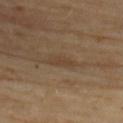Imaged during a routine full-body skin examination; the lesion was not biopsied and no histopathology is available.
Measured at roughly 3 mm in maximum diameter.
The lesion is on the upper back.
A roughly 15 mm field-of-view crop from a total-body skin photograph.
Captured under cross-polarized illumination.
A female patient, about 45 years old.
The total-body-photography lesion software estimated a border-irregularity rating of about 3.5/10 and radial color variation of about 0. And it measured a nevus-likeness score of about 5/100 and a detector confidence of about 60 out of 100 that the crop contains a lesion.